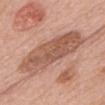Q: Was a biopsy performed?
A: no biopsy performed (imaged during a skin exam)
Q: What is the anatomic site?
A: the head or neck
Q: How was this image acquired?
A: total-body-photography crop, ~15 mm field of view
Q: What is the lesion's diameter?
A: about 10 mm
Q: Illumination type?
A: white-light illumination
Q: Patient demographics?
A: female, about 60 years old
Q: Automated lesion metrics?
A: a lesion area of about 29 mm², an eccentricity of roughly 0.95, and two-axis asymmetry of about 0.15; border irregularity of about 3 on a 0–10 scale; a nevus-likeness score of about 0/100 and lesion-presence confidence of about 100/100